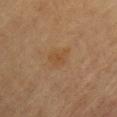Impression:
Captured during whole-body skin photography for melanoma surveillance; the lesion was not biopsied.
Image and clinical context:
Located on the chest. A female subject, in their mid-60s. This is a cross-polarized tile. Cropped from a whole-body photographic skin survey; the tile spans about 15 mm.